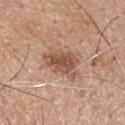workup: imaged on a skin check; not biopsied | subject: male, aged approximately 75 | tile lighting: white-light | image: 15 mm crop, total-body photography | anatomic site: the upper back.